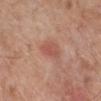<case>
  <biopsy_status>not biopsied; imaged during a skin examination</biopsy_status>
  <patient>
    <sex>male</sex>
    <age_approx>80</age_approx>
  </patient>
  <site>right lower leg</site>
  <image>
    <source>total-body photography crop</source>
    <field_of_view_mm>15</field_of_view_mm>
  </image>
  <lighting>white-light</lighting>
  <lesion_size>
    <long_diameter_mm_approx>3.0</long_diameter_mm_approx>
  </lesion_size>
  <automated_metrics>
    <area_mm2_approx>4.0</area_mm2_approx>
    <eccentricity>0.85</eccentricity>
    <shape_asymmetry>0.3</shape_asymmetry>
    <cielab_L>53</cielab_L>
    <cielab_a>26</cielab_a>
    <cielab_b>29</cielab_b>
    <vs_skin_darker_L>8.0</vs_skin_darker_L>
    <border_irregularity_0_10>2.5</border_irregularity_0_10>
    <color_variation_0_10>3.0</color_variation_0_10>
    <peripheral_color_asymmetry>1.0</peripheral_color_asymmetry>
  </automated_metrics>
</case>